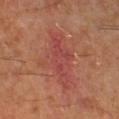Assessment:
Part of a total-body skin-imaging series; this lesion was reviewed on a skin check and was not flagged for biopsy.
Context:
On the right lower leg. The lesion-visualizer software estimated a lesion area of about 21 mm² and a symmetry-axis asymmetry near 0.35. The software also gave about 6 CIELAB-L* units darker than the surrounding skin. The analysis additionally found a classifier nevus-likeness of about 0/100. The tile uses cross-polarized illumination. Cropped from a whole-body photographic skin survey; the tile spans about 15 mm. The patient is a male aged approximately 60. Approximately 7 mm at its widest.